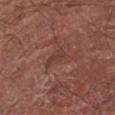Context: Longest diameter approximately 3 mm. A 15 mm crop from a total-body photograph taken for skin-cancer surveillance. From the right forearm. A female subject aged 78 to 82. Imaged with white-light lighting.